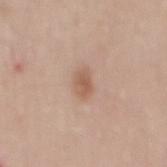Q: Is there a histopathology result?
A: total-body-photography surveillance lesion; no biopsy
Q: Lesion location?
A: the mid back
Q: What are the patient's age and sex?
A: male, about 50 years old
Q: What is the imaging modality?
A: total-body-photography crop, ~15 mm field of view